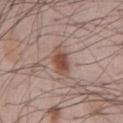Clinical impression:
This lesion was catalogued during total-body skin photography and was not selected for biopsy.
Image and clinical context:
A 15 mm close-up tile from a total-body photography series done for melanoma screening. A male subject, aged 63–67. Measured at roughly 3.5 mm in maximum diameter. Located on the chest. An algorithmic analysis of the crop reported a lesion area of about 6 mm², an outline eccentricity of about 0.75 (0 = round, 1 = elongated), and a symmetry-axis asymmetry near 0.15. The analysis additionally found roughly 11 lightness units darker than nearby skin and a normalized border contrast of about 9. It also reported a border-irregularity rating of about 1.5/10, a within-lesion color-variation index near 4.5/10, and peripheral color asymmetry of about 1.5. The analysis additionally found a classifier nevus-likeness of about 90/100 and a lesion-detection confidence of about 100/100. This is a white-light tile.